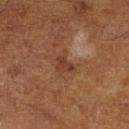Q: Was this lesion biopsied?
A: catalogued during a skin exam; not biopsied
Q: Lesion location?
A: the leg
Q: How was this image acquired?
A: ~15 mm tile from a whole-body skin photo
Q: How was the tile lit?
A: cross-polarized illumination
Q: What is the lesion's diameter?
A: ~2.5 mm (longest diameter)
Q: Who is the patient?
A: male, roughly 65 years of age